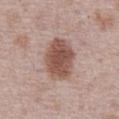Part of a total-body skin-imaging series; this lesion was reviewed on a skin check and was not flagged for biopsy.